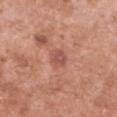{
  "biopsy_status": "not biopsied; imaged during a skin examination",
  "lighting": "white-light",
  "patient": {
    "sex": "female",
    "age_approx": 50
  },
  "image": {
    "source": "total-body photography crop",
    "field_of_view_mm": 15
  },
  "automated_metrics": {
    "nevus_likeness_0_100": 0,
    "lesion_detection_confidence_0_100": 100
  },
  "site": "front of the torso"
}